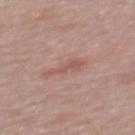follow-up: total-body-photography surveillance lesion; no biopsy.